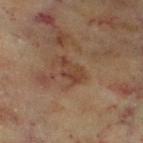{
  "biopsy_status": "not biopsied; imaged during a skin examination",
  "lesion_size": {
    "long_diameter_mm_approx": 3.0
  },
  "patient": {
    "sex": "female",
    "age_approx": 60
  },
  "image": {
    "source": "total-body photography crop",
    "field_of_view_mm": 15
  },
  "lighting": "cross-polarized",
  "site": "left lower leg"
}